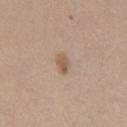Recorded during total-body skin imaging; not selected for excision or biopsy.
Approximately 2.5 mm at its widest.
On the mid back.
A female subject aged approximately 40.
The tile uses white-light illumination.
A roughly 15 mm field-of-view crop from a total-body skin photograph.